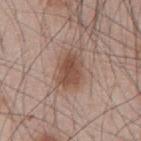Q: Was a biopsy performed?
A: total-body-photography surveillance lesion; no biopsy
Q: Lesion size?
A: about 4 mm
Q: What kind of image is this?
A: total-body-photography crop, ~15 mm field of view
Q: Lesion location?
A: the abdomen
Q: What did automated image analysis measure?
A: a mean CIELAB color near L≈49 a*≈19 b*≈27; an automated nevus-likeness rating near 95 out of 100 and a detector confidence of about 100 out of 100 that the crop contains a lesion
Q: What are the patient's age and sex?
A: male, roughly 45 years of age